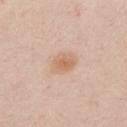The lesion was photographed on a routine skin check and not biopsied; there is no pathology result.
Imaged with white-light lighting.
The lesion is located on the chest.
Measured at roughly 3 mm in maximum diameter.
A male subject, roughly 40 years of age.
The total-body-photography lesion software estimated an area of roughly 6.5 mm² and an outline eccentricity of about 0.7 (0 = round, 1 = elongated). And it measured a classifier nevus-likeness of about 60/100 and lesion-presence confidence of about 100/100.
A lesion tile, about 15 mm wide, cut from a 3D total-body photograph.